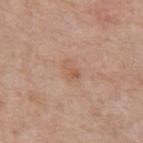Imaged during a routine full-body skin examination; the lesion was not biopsied and no histopathology is available.
A 15 mm close-up extracted from a 3D total-body photography capture.
A male subject approximately 70 years of age.
The lesion's longest dimension is about 2.5 mm.
Imaged with white-light lighting.
From the front of the torso.